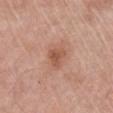Captured during whole-body skin photography for melanoma surveillance; the lesion was not biopsied.
The lesion is located on the front of the torso.
A region of skin cropped from a whole-body photographic capture, roughly 15 mm wide.
Automated image analysis of the tile measured an eccentricity of roughly 0.65 and two-axis asymmetry of about 0.4. The software also gave internal color variation of about 2.5 on a 0–10 scale and a peripheral color-asymmetry measure near 1. And it measured lesion-presence confidence of about 100/100.
A male patient about 65 years old.